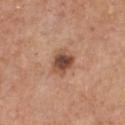The lesion was photographed on a routine skin check and not biopsied; there is no pathology result. A 15 mm close-up tile from a total-body photography series done for melanoma screening. This is a white-light tile. The lesion is located on the chest. Longest diameter approximately 3 mm. A male subject aged around 60.